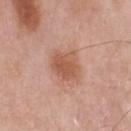Acquisition and patient details:
About 3.5 mm across. This image is a 15 mm lesion crop taken from a total-body photograph. This is a white-light tile. The lesion is on the chest. An algorithmic analysis of the crop reported a mean CIELAB color near L≈56 a*≈24 b*≈31, roughly 10 lightness units darker than nearby skin, and a normalized lesion–skin contrast near 7.5. A male patient about 55 years old.